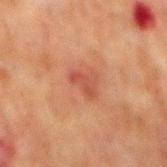<tbp_lesion>
  <biopsy_status>not biopsied; imaged during a skin examination</biopsy_status>
  <site>mid back</site>
  <lesion_size>
    <long_diameter_mm_approx>3.0</long_diameter_mm_approx>
  </lesion_size>
  <lighting>cross-polarized</lighting>
  <automated_metrics>
    <area_mm2_approx>2.5</area_mm2_approx>
    <eccentricity>0.9</eccentricity>
    <shape_asymmetry>0.45</shape_asymmetry>
    <color_variation_0_10>0.0</color_variation_0_10>
    <peripheral_color_asymmetry>0.0</peripheral_color_asymmetry>
  </automated_metrics>
  <image>
    <source>total-body photography crop</source>
    <field_of_view_mm>15</field_of_view_mm>
  </image>
  <patient>
    <sex>male</sex>
    <age_approx>60</age_approx>
  </patient>
</tbp_lesion>